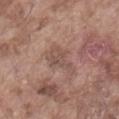No biopsy was performed on this lesion — it was imaged during a full skin examination and was not determined to be concerning. A 15 mm close-up tile from a total-body photography series done for melanoma screening. The patient is a male aged around 75. Approximately 4 mm at its widest. The tile uses white-light illumination. Located on the abdomen.